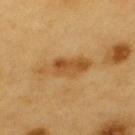– notes: no biopsy performed (imaged during a skin exam)
– patient: male, aged around 60
– image: ~15 mm crop, total-body skin-cancer survey
– location: the upper back
– diameter: ≈6 mm